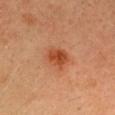Imaged during a routine full-body skin examination; the lesion was not biopsied and no histopathology is available.
This is a cross-polarized tile.
Approximately 3 mm at its widest.
The patient is a male in their 40s.
This image is a 15 mm lesion crop taken from a total-body photograph.
Automated image analysis of the tile measured an area of roughly 6.5 mm² and a shape eccentricity near 0.45. And it measured a lesion color around L≈43 a*≈27 b*≈35 in CIELAB, about 10 CIELAB-L* units darker than the surrounding skin, and a normalized lesion–skin contrast near 8.5. The software also gave internal color variation of about 4 on a 0–10 scale and a peripheral color-asymmetry measure near 1.5. And it measured lesion-presence confidence of about 100/100.
From the head or neck.